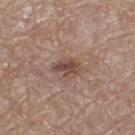The lesion was photographed on a routine skin check and not biopsied; there is no pathology result.
A female patient, about 55 years old.
The lesion is located on the left thigh.
A 15 mm close-up tile from a total-body photography series done for melanoma screening.